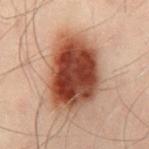Clinical impression: Imaged during a routine full-body skin examination; the lesion was not biopsied and no histopathology is available.